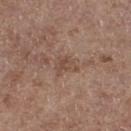workup=imaged on a skin check; not biopsied
acquisition=total-body-photography crop, ~15 mm field of view
lighting=white-light
location=the right thigh
subject=female, in their mid-60s